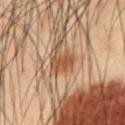| field | value |
|---|---|
| workup | imaged on a skin check; not biopsied |
| acquisition | 15 mm crop, total-body photography |
| diameter | ~3 mm (longest diameter) |
| illumination | cross-polarized illumination |
| body site | the abdomen |
| subject | male, approximately 55 years of age |
| automated lesion analysis | an area of roughly 5 mm², a shape eccentricity near 0.65, and a shape-asymmetry score of about 0.25 (0 = symmetric); a detector confidence of about 100 out of 100 that the crop contains a lesion |